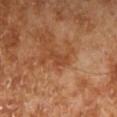Longest diameter approximately 2.5 mm.
The lesion is on the left lower leg.
A male patient aged approximately 70.
Captured under cross-polarized illumination.
A close-up tile cropped from a whole-body skin photograph, about 15 mm across.